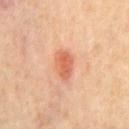lesion_size:
  long_diameter_mm_approx: 3.5
patient:
  sex: male
  age_approx: 70
site: chest
lighting: cross-polarized
automated_metrics:
  border_irregularity_0_10: 1.5
  color_variation_0_10: 3.0
  peripheral_color_asymmetry: 1.0
image:
  source: total-body photography crop
  field_of_view_mm: 15